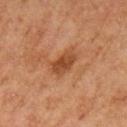biopsy status: imaged on a skin check; not biopsied
imaging modality: ~15 mm tile from a whole-body skin photo
automated metrics: an area of roughly 6.5 mm², an outline eccentricity of about 0.8 (0 = round, 1 = elongated), and two-axis asymmetry of about 0.25; roughly 9 lightness units darker than nearby skin; a lesion-detection confidence of about 100/100
location: the left upper arm
subject: female, in their 60s
lighting: cross-polarized